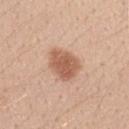notes = imaged on a skin check; not biopsied
automated metrics = a footprint of about 11 mm², an eccentricity of roughly 0.6, and two-axis asymmetry of about 0.2; a lesion color around L≈58 a*≈22 b*≈31 in CIELAB and a lesion–skin lightness drop of about 13; a border-irregularity index near 2/10 and a peripheral color-asymmetry measure near 1; a detector confidence of about 100 out of 100 that the crop contains a lesion
size = ~4 mm (longest diameter)
lighting = white-light illumination
acquisition = 15 mm crop, total-body photography
anatomic site = the arm
subject = female, approximately 25 years of age